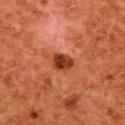Case summary:
* workup — catalogued during a skin exam; not biopsied
* lighting — cross-polarized
* image source — ~15 mm tile from a whole-body skin photo
* TBP lesion metrics — a footprint of about 5 mm², a shape eccentricity near 0.65, and a symmetry-axis asymmetry near 0.15; border irregularity of about 1 on a 0–10 scale and radial color variation of about 1.5; a nevus-likeness score of about 70/100
* subject — female, aged around 50
* location — the upper back